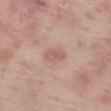notes = total-body-photography surveillance lesion; no biopsy
subject = male, aged around 65
anatomic site = the left thigh
acquisition = total-body-photography crop, ~15 mm field of view
lesion size = ~2.5 mm (longest diameter)
tile lighting = white-light